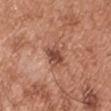tile lighting=white-light | anatomic site=the chest | diameter=about 3 mm | patient=male, about 55 years old | TBP lesion metrics=an average lesion color of about L≈49 a*≈23 b*≈29 (CIELAB), roughly 11 lightness units darker than nearby skin, and a normalized lesion–skin contrast near 7.5; a border-irregularity rating of about 2.5/10 and peripheral color asymmetry of about 1.5; an automated nevus-likeness rating near 75 out of 100 and lesion-presence confidence of about 100/100 | imaging modality=15 mm crop, total-body photography.